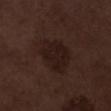{"biopsy_status": "not biopsied; imaged during a skin examination", "patient": {"sex": "male", "age_approx": 70}, "site": "left lower leg", "lesion_size": {"long_diameter_mm_approx": 5.0}, "image": {"source": "total-body photography crop", "field_of_view_mm": 15}}